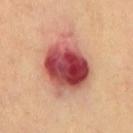Impression: The lesion was tiled from a total-body skin photograph and was not biopsied. Context: A male patient about 70 years old. This image is a 15 mm lesion crop taken from a total-body photograph. The lesion-visualizer software estimated a mean CIELAB color near L≈49 a*≈30 b*≈26 and a normalized lesion–skin contrast near 13. The software also gave internal color variation of about 10 on a 0–10 scale and radial color variation of about 3.5. It also reported a nevus-likeness score of about 0/100 and lesion-presence confidence of about 100/100. From the chest. Measured at roughly 7 mm in maximum diameter.